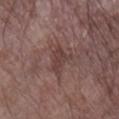Impression: Recorded during total-body skin imaging; not selected for excision or biopsy. Acquisition and patient details: Approximately 3 mm at its widest. This is a white-light tile. From the right lower leg. The patient is a male aged 78 to 82. This image is a 15 mm lesion crop taken from a total-body photograph.